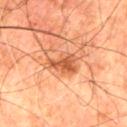Recorded during total-body skin imaging; not selected for excision or biopsy.
A 15 mm close-up tile from a total-body photography series done for melanoma screening.
The subject is a male in their 80s.
Approximately 4 mm at its widest.
Automated image analysis of the tile measured an average lesion color of about L≈45 a*≈24 b*≈33 (CIELAB), roughly 10 lightness units darker than nearby skin, and a normalized lesion–skin contrast near 7.5. And it measured a nevus-likeness score of about 60/100.
From the left thigh.